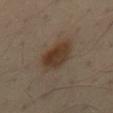follow-up: imaged on a skin check; not biopsied
location: the lower back
lighting: cross-polarized illumination
acquisition: ~15 mm tile from a whole-body skin photo
subject: male, in their mid-50s